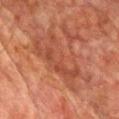Assessment:
Recorded during total-body skin imaging; not selected for excision or biopsy.
Clinical summary:
Imaged with cross-polarized lighting. Located on the chest. The lesion-visualizer software estimated an area of roughly 24 mm² and two-axis asymmetry of about 0.35. It also reported a lesion color around L≈41 a*≈24 b*≈28 in CIELAB, about 6 CIELAB-L* units darker than the surrounding skin, and a normalized lesion–skin contrast near 5.5. A male patient in their mid- to late 70s. Approximately 9 mm at its widest. A 15 mm close-up tile from a total-body photography series done for melanoma screening.